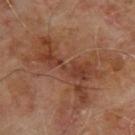* biopsy status: imaged on a skin check; not biopsied
* lighting: cross-polarized
* patient: male, aged 63–67
* site: the upper back
* automated lesion analysis: a footprint of about 20 mm², an outline eccentricity of about 0.9 (0 = round, 1 = elongated), and a symmetry-axis asymmetry near 0.5; an average lesion color of about L≈38 a*≈22 b*≈29 (CIELAB) and a normalized border contrast of about 7; internal color variation of about 4 on a 0–10 scale and peripheral color asymmetry of about 1; a nevus-likeness score of about 0/100 and a detector confidence of about 100 out of 100 that the crop contains a lesion
* size: about 9 mm
* imaging modality: ~15 mm tile from a whole-body skin photo